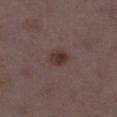<record>
<biopsy_status>not biopsied; imaged during a skin examination</biopsy_status>
<image>
  <source>total-body photography crop</source>
  <field_of_view_mm>15</field_of_view_mm>
</image>
<automated_metrics>
  <cielab_L>34</cielab_L>
  <cielab_a>16</cielab_a>
  <cielab_b>19</cielab_b>
  <vs_skin_darker_L>8.0</vs_skin_darker_L>
  <vs_skin_contrast_norm>8.5</vs_skin_contrast_norm>
  <border_irregularity_0_10>1.5</border_irregularity_0_10>
  <color_variation_0_10>3.0</color_variation_0_10>
  <peripheral_color_asymmetry>1.0</peripheral_color_asymmetry>
  <nevus_likeness_0_100>100</nevus_likeness_0_100>
  <lesion_detection_confidence_0_100>100</lesion_detection_confidence_0_100>
</automated_metrics>
<lighting>white-light</lighting>
<lesion_size>
  <long_diameter_mm_approx>2.5</long_diameter_mm_approx>
</lesion_size>
<patient>
  <sex>female</sex>
  <age_approx>35</age_approx>
</patient>
<site>leg</site>
</record>